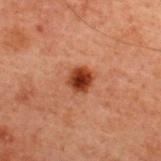biopsy status: imaged on a skin check; not biopsied
size: ≈2.5 mm
imaging modality: 15 mm crop, total-body photography
body site: the upper back
image-analysis metrics: a classifier nevus-likeness of about 100/100 and a detector confidence of about 100 out of 100 that the crop contains a lesion
patient: male, aged around 60
tile lighting: cross-polarized illumination